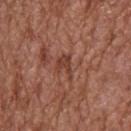workup = no biopsy performed (imaged during a skin exam) | image source = ~15 mm tile from a whole-body skin photo | body site = the back | subject = male, in their mid- to late 70s.